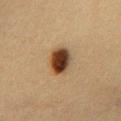<record>
<biopsy_status>not biopsied; imaged during a skin examination</biopsy_status>
<site>chest</site>
<patient>
  <sex>female</sex>
  <age_approx>40</age_approx>
</patient>
<image>
  <source>total-body photography crop</source>
  <field_of_view_mm>15</field_of_view_mm>
</image>
</record>